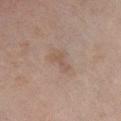Impression:
The lesion was photographed on a routine skin check and not biopsied; there is no pathology result.
Image and clinical context:
A female patient, aged approximately 60. From the right lower leg. Cropped from a whole-body photographic skin survey; the tile spans about 15 mm. The tile uses cross-polarized illumination.